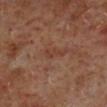Findings:
– notes: no biopsy performed (imaged during a skin exam)
– tile lighting: cross-polarized
– patient: male, aged 58–62
– image: total-body-photography crop, ~15 mm field of view
– site: the leg
– diameter: ~2.5 mm (longest diameter)
– image-analysis metrics: a footprint of about 2.5 mm², a shape eccentricity near 0.9, and two-axis asymmetry of about 0.45